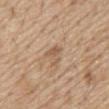biopsy_status: not biopsied; imaged during a skin examination
image:
  source: total-body photography crop
  field_of_view_mm: 15
site: abdomen
lighting: white-light
automated_metrics:
  cielab_L: 57
  cielab_a: 17
  cielab_b: 32
  vs_skin_darker_L: 8.0
  vs_skin_contrast_norm: 5.5
  border_irregularity_0_10: 5.5
  color_variation_0_10: 1.0
  peripheral_color_asymmetry: 0.0
  nevus_likeness_0_100: 0
  lesion_detection_confidence_0_100: 95
patient:
  sex: male
  age_approx: 70
lesion_size:
  long_diameter_mm_approx: 2.5